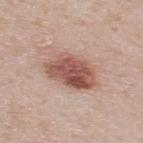{
  "biopsy_status": "not biopsied; imaged during a skin examination",
  "lighting": "white-light",
  "automated_metrics": {
    "area_mm2_approx": 16.0,
    "shape_asymmetry": 0.2,
    "vs_skin_darker_L": 14.0,
    "vs_skin_contrast_norm": 9.5,
    "color_variation_0_10": 6.0,
    "peripheral_color_asymmetry": 2.0,
    "nevus_likeness_0_100": 100,
    "lesion_detection_confidence_0_100": 100
  },
  "patient": {
    "sex": "male",
    "age_approx": 50
  },
  "lesion_size": {
    "long_diameter_mm_approx": 6.0
  },
  "image": {
    "source": "total-body photography crop",
    "field_of_view_mm": 15
  },
  "site": "upper back"
}